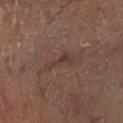- notes: catalogued during a skin exam; not biopsied
- location: the right lower leg
- lighting: cross-polarized illumination
- subject: male, aged around 65
- imaging modality: ~15 mm crop, total-body skin-cancer survey